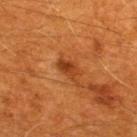Clinical impression:
The lesion was photographed on a routine skin check and not biopsied; there is no pathology result.
Acquisition and patient details:
The lesion is on the back. The subject is a male in their 60s. This image is a 15 mm lesion crop taken from a total-body photograph.